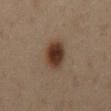Impression: Captured during whole-body skin photography for melanoma surveillance; the lesion was not biopsied. Background: The lesion-visualizer software estimated a lesion color around L≈32 a*≈16 b*≈25 in CIELAB and a lesion-to-skin contrast of about 12 (normalized; higher = more distinct). The software also gave a detector confidence of about 100 out of 100 that the crop contains a lesion. On the abdomen. The patient is a male about 60 years old. This image is a 15 mm lesion crop taken from a total-body photograph. Imaged with cross-polarized lighting.